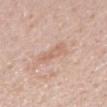Clinical impression:
Imaged during a routine full-body skin examination; the lesion was not biopsied and no histopathology is available.
Image and clinical context:
An algorithmic analysis of the crop reported a lesion area of about 3 mm² and a shape-asymmetry score of about 0.4 (0 = symmetric). The software also gave a border-irregularity index near 4.5/10 and a peripheral color-asymmetry measure near 0.5. And it measured an automated nevus-likeness rating near 0 out of 100. Longest diameter approximately 2.5 mm. From the right forearm. The tile uses white-light illumination. A 15 mm crop from a total-body photograph taken for skin-cancer surveillance. A female patient, in their 30s.